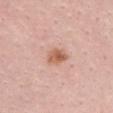The lesion was tiled from a total-body skin photograph and was not biopsied.
From the upper back.
Imaged with white-light lighting.
A lesion tile, about 15 mm wide, cut from a 3D total-body photograph.
A female subject aged 23 to 27.